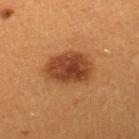biopsy_status: not biopsied; imaged during a skin examination